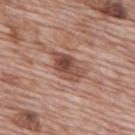The lesion was tiled from a total-body skin photograph and was not biopsied.
Approximately 4.5 mm at its widest.
From the back.
Automated tile analysis of the lesion measured a footprint of about 9 mm², an outline eccentricity of about 0.8 (0 = round, 1 = elongated), and two-axis asymmetry of about 0.25. The software also gave an average lesion color of about L≈49 a*≈22 b*≈26 (CIELAB), roughly 12 lightness units darker than nearby skin, and a normalized border contrast of about 8.5. And it measured a classifier nevus-likeness of about 30/100 and lesion-presence confidence of about 100/100.
The patient is a male roughly 70 years of age.
A close-up tile cropped from a whole-body skin photograph, about 15 mm across.
Captured under white-light illumination.